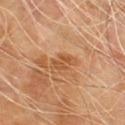Part of a total-body skin-imaging series; this lesion was reviewed on a skin check and was not flagged for biopsy. A roughly 15 mm field-of-view crop from a total-body skin photograph. From the chest. An algorithmic analysis of the crop reported a footprint of about 4.5 mm² and a shape-asymmetry score of about 0.35 (0 = symmetric). The software also gave a border-irregularity rating of about 5/10, a within-lesion color-variation index near 0.5/10, and a peripheral color-asymmetry measure near 0. A male patient, aged 68 to 72. Approximately 3 mm at its widest. Imaged with cross-polarized lighting.